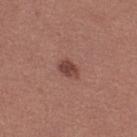Case summary:
* follow-up — no biopsy performed (imaged during a skin exam)
* illumination — white-light
* image source — ~15 mm tile from a whole-body skin photo
* patient — female, in their mid- to late 20s
* automated metrics — a mean CIELAB color near L≈43 a*≈24 b*≈24, roughly 11 lightness units darker than nearby skin, and a normalized border contrast of about 8.5; internal color variation of about 3 on a 0–10 scale and radial color variation of about 1; a classifier nevus-likeness of about 85/100 and a lesion-detection confidence of about 100/100
* lesion diameter — ≈3 mm
* location — the right thigh